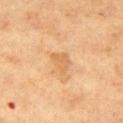Part of a total-body skin-imaging series; this lesion was reviewed on a skin check and was not flagged for biopsy.
Located on the right thigh.
A 15 mm crop from a total-body photograph taken for skin-cancer surveillance.
A female subject roughly 40 years of age.